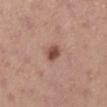Clinical impression:
This lesion was catalogued during total-body skin photography and was not selected for biopsy.
Image and clinical context:
The subject is a female in their mid- to late 20s. The lesion is on the right lower leg. Imaged with white-light lighting. A roughly 15 mm field-of-view crop from a total-body skin photograph.